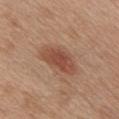No biopsy was performed on this lesion — it was imaged during a full skin examination and was not determined to be concerning. A region of skin cropped from a whole-body photographic capture, roughly 15 mm wide. Located on the front of the torso. A female patient aged approximately 40. Automated image analysis of the tile measured a footprint of about 11 mm², an outline eccentricity of about 0.85 (0 = round, 1 = elongated), and a shape-asymmetry score of about 0.25 (0 = symmetric). It also reported a mean CIELAB color near L≈49 a*≈23 b*≈30, roughly 10 lightness units darker than nearby skin, and a normalized lesion–skin contrast near 7.5. And it measured a border-irregularity index near 2.5/10 and peripheral color asymmetry of about 1. Measured at roughly 5 mm in maximum diameter.